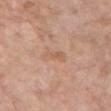Captured during whole-body skin photography for melanoma surveillance; the lesion was not biopsied. On the chest. The tile uses white-light illumination. A region of skin cropped from a whole-body photographic capture, roughly 15 mm wide. A female subject aged around 75. An algorithmic analysis of the crop reported an area of roughly 3.5 mm², an eccentricity of roughly 0.9, and a shape-asymmetry score of about 0.3 (0 = symmetric). The software also gave a classifier nevus-likeness of about 0/100 and a lesion-detection confidence of about 100/100. Approximately 3 mm at its widest.